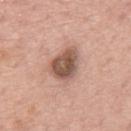| key | value |
|---|---|
| patient | male, approximately 75 years of age |
| location | the mid back |
| automated metrics | a lesion area of about 11 mm², an eccentricity of roughly 0.75, and a symmetry-axis asymmetry near 0.3; a peripheral color-asymmetry measure near 1.5; a classifier nevus-likeness of about 10/100 and a lesion-detection confidence of about 100/100 |
| diameter | ≈4.5 mm |
| image source | ~15 mm crop, total-body skin-cancer survey |